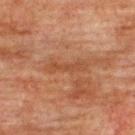Assessment:
Part of a total-body skin-imaging series; this lesion was reviewed on a skin check and was not flagged for biopsy.
Image and clinical context:
The tile uses cross-polarized illumination. Longest diameter approximately 4.5 mm. The lesion is on the upper back. A roughly 15 mm field-of-view crop from a total-body skin photograph. The subject is a male roughly 75 years of age.